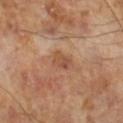Clinical impression:
No biopsy was performed on this lesion — it was imaged during a full skin examination and was not determined to be concerning.
Background:
A male subject about 65 years old. Longest diameter approximately 2.5 mm. A 15 mm close-up tile from a total-body photography series done for melanoma screening. Captured under cross-polarized illumination.